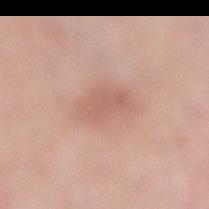Captured during whole-body skin photography for melanoma surveillance; the lesion was not biopsied. The patient is a female aged 53 to 57. On the right lower leg. The recorded lesion diameter is about 4 mm. Imaged with white-light lighting. Cropped from a whole-body photographic skin survey; the tile spans about 15 mm.